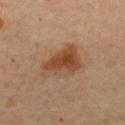{
  "biopsy_status": "not biopsied; imaged during a skin examination",
  "site": "upper back",
  "image": {
    "source": "total-body photography crop",
    "field_of_view_mm": 15
  },
  "patient": {
    "sex": "female",
    "age_approx": 45
  }
}